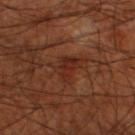{
  "biopsy_status": "not biopsied; imaged during a skin examination",
  "lesion_size": {
    "long_diameter_mm_approx": 3.0
  },
  "site": "left lower leg",
  "lighting": "cross-polarized",
  "patient": {
    "sex": "male",
    "age_approx": 70
  },
  "image": {
    "source": "total-body photography crop",
    "field_of_view_mm": 15
  }
}